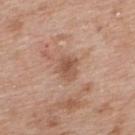notes: total-body-photography surveillance lesion; no biopsy
tile lighting: white-light
image-analysis metrics: an area of roughly 6 mm², an outline eccentricity of about 0.5 (0 = round, 1 = elongated), and a symmetry-axis asymmetry near 0.25; a classifier nevus-likeness of about 0/100 and a lesion-detection confidence of about 100/100
lesion size: about 3 mm
acquisition: total-body-photography crop, ~15 mm field of view
subject: female, aged 38 to 42
site: the upper back